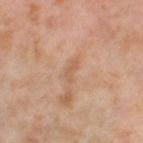<record>
<biopsy_status>not biopsied; imaged during a skin examination</biopsy_status>
<lesion_size>
  <long_diameter_mm_approx>2.5</long_diameter_mm_approx>
</lesion_size>
<automated_metrics>
  <cielab_L>59</cielab_L>
  <cielab_a>21</cielab_a>
  <cielab_b>34</cielab_b>
  <vs_skin_darker_L>7.0</vs_skin_darker_L>
  <vs_skin_contrast_norm>5.0</vs_skin_contrast_norm>
  <nevus_likeness_0_100>0</nevus_likeness_0_100>
</automated_metrics>
<site>right thigh</site>
<patient>
  <sex>female</sex>
  <age_approx>55</age_approx>
</patient>
<lighting>cross-polarized</lighting>
<image>
  <source>total-body photography crop</source>
  <field_of_view_mm>15</field_of_view_mm>
</image>
</record>